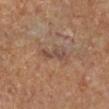{"biopsy_status": "not biopsied; imaged during a skin examination", "patient": {"sex": "male", "age_approx": 60}, "site": "left lower leg", "image": {"source": "total-body photography crop", "field_of_view_mm": 15}}